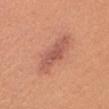{
  "site": "head or neck",
  "lesion_size": {
    "long_diameter_mm_approx": 6.0
  },
  "automated_metrics": {
    "area_mm2_approx": 12.0,
    "eccentricity": 0.95,
    "nevus_likeness_0_100": 15,
    "lesion_detection_confidence_0_100": 100
  },
  "image": {
    "source": "total-body photography crop",
    "field_of_view_mm": 15
  },
  "lighting": "white-light",
  "patient": {
    "sex": "female",
    "age_approx": 25
  }
}